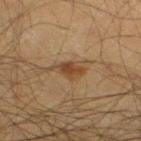Part of a total-body skin-imaging series; this lesion was reviewed on a skin check and was not flagged for biopsy.
On the leg.
A male patient, aged approximately 50.
The total-body-photography lesion software estimated an area of roughly 4.5 mm² and a shape eccentricity near 0.8. It also reported an average lesion color of about L≈35 a*≈15 b*≈28 (CIELAB) and a normalized border contrast of about 8. The analysis additionally found border irregularity of about 2.5 on a 0–10 scale and peripheral color asymmetry of about 1. The analysis additionally found a nevus-likeness score of about 60/100 and a lesion-detection confidence of about 100/100.
A close-up tile cropped from a whole-body skin photograph, about 15 mm across.